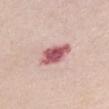From the chest. The recorded lesion diameter is about 4.5 mm. A female patient aged approximately 60. Imaged with white-light lighting. A close-up tile cropped from a whole-body skin photograph, about 15 mm across.